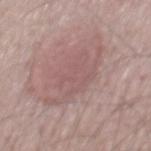notes = imaged on a skin check; not biopsied
subject = male, aged 63 to 67
image source = ~15 mm crop, total-body skin-cancer survey
site = the mid back
image-analysis metrics = a lesion color around L≈55 a*≈20 b*≈19 in CIELAB and about 7 CIELAB-L* units darker than the surrounding skin; internal color variation of about 2 on a 0–10 scale; a nevus-likeness score of about 10/100
illumination = white-light